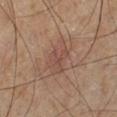Recorded during total-body skin imaging; not selected for excision or biopsy. Longest diameter approximately 4.5 mm. On the leg. This is a cross-polarized tile. The patient is a male roughly 65 years of age. A 15 mm close-up tile from a total-body photography series done for melanoma screening.